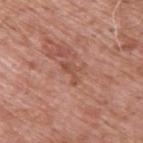lighting: white-light illumination | image: ~15 mm crop, total-body skin-cancer survey | lesion size: about 3 mm | anatomic site: the back | subject: male, aged approximately 60.